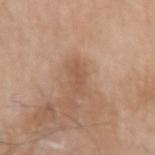<tbp_lesion>
<lesion_size>
  <long_diameter_mm_approx>3.0</long_diameter_mm_approx>
</lesion_size>
<lighting>white-light</lighting>
<automated_metrics>
  <area_mm2_approx>4.5</area_mm2_approx>
  <shape_asymmetry>0.25</shape_asymmetry>
  <color_variation_0_10>0.5</color_variation_0_10>
  <peripheral_color_asymmetry>0.0</peripheral_color_asymmetry>
  <nevus_likeness_0_100>0</nevus_likeness_0_100>
  <lesion_detection_confidence_0_100>100</lesion_detection_confidence_0_100>
</automated_metrics>
<patient>
  <sex>male</sex>
  <age_approx>75</age_approx>
</patient>
<site>left upper arm</site>
<image>
  <source>total-body photography crop</source>
  <field_of_view_mm>15</field_of_view_mm>
</image>
</tbp_lesion>